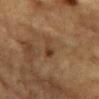| field | value |
|---|---|
| notes | total-body-photography surveillance lesion; no biopsy |
| lesion size | about 3 mm |
| acquisition | ~15 mm crop, total-body skin-cancer survey |
| subject | male, aged 83 or older |
| tile lighting | cross-polarized |
| site | the mid back |